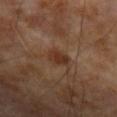<lesion>
<biopsy_status>not biopsied; imaged during a skin examination</biopsy_status>
<automated_metrics>
  <area_mm2_approx>4.5</area_mm2_approx>
  <eccentricity>0.55</eccentricity>
  <shape_asymmetry>0.25</shape_asymmetry>
</automated_metrics>
<image>
  <source>total-body photography crop</source>
  <field_of_view_mm>15</field_of_view_mm>
</image>
<site>left forearm</site>
<patient>
  <sex>male</sex>
  <age_approx>70</age_approx>
</patient>
<lesion_size>
  <long_diameter_mm_approx>2.5</long_diameter_mm_approx>
</lesion_size>
</lesion>